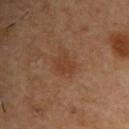<record>
<biopsy_status>not biopsied; imaged during a skin examination</biopsy_status>
<lesion_size>
  <long_diameter_mm_approx>3.0</long_diameter_mm_approx>
</lesion_size>
<automated_metrics>
  <area_mm2_approx>5.0</area_mm2_approx>
  <eccentricity>0.65</eccentricity>
  <shape_asymmetry>0.35</shape_asymmetry>
  <border_irregularity_0_10>3.5</border_irregularity_0_10>
  <color_variation_0_10>2.0</color_variation_0_10>
  <peripheral_color_asymmetry>1.0</peripheral_color_asymmetry>
  <nevus_likeness_0_100>15</nevus_likeness_0_100>
  <lesion_detection_confidence_0_100>100</lesion_detection_confidence_0_100>
</automated_metrics>
<site>right upper arm</site>
<lighting>cross-polarized</lighting>
<patient>
  <sex>male</sex>
  <age_approx>55</age_approx>
</patient>
<image>
  <source>total-body photography crop</source>
  <field_of_view_mm>15</field_of_view_mm>
</image>
</record>